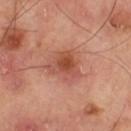Notes:
– follow-up — imaged on a skin check; not biopsied
– lesion size — ~4 mm (longest diameter)
– automated lesion analysis — a lesion color around L≈52 a*≈28 b*≈32 in CIELAB, roughly 10 lightness units darker than nearby skin, and a lesion-to-skin contrast of about 7.5 (normalized; higher = more distinct); a border-irregularity index near 3.5/10, a within-lesion color-variation index near 6/10, and peripheral color asymmetry of about 1.5
– imaging modality — 15 mm crop, total-body photography
– site — the right thigh